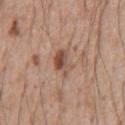Notes:
• workup — no biopsy performed (imaged during a skin exam)
• location — the front of the torso
• subject — male, in their mid- to late 50s
• imaging modality — ~15 mm crop, total-body skin-cancer survey